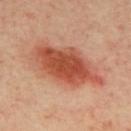Q: Is there a histopathology result?
A: catalogued during a skin exam; not biopsied
Q: Who is the patient?
A: male, aged 38 to 42
Q: What did automated image analysis measure?
A: an average lesion color of about L≈53 a*≈30 b*≈34 (CIELAB), about 14 CIELAB-L* units darker than the surrounding skin, and a normalized border contrast of about 9.5; a nevus-likeness score of about 100/100 and lesion-presence confidence of about 100/100
Q: What is the lesion's diameter?
A: ≈9.5 mm
Q: What is the imaging modality?
A: ~15 mm tile from a whole-body skin photo
Q: What is the anatomic site?
A: the upper back